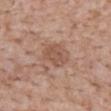Captured during whole-body skin photography for melanoma surveillance; the lesion was not biopsied. A lesion tile, about 15 mm wide, cut from a 3D total-body photograph. On the mid back. A male subject, about 45 years old. Captured under white-light illumination. Approximately 4 mm at its widest.